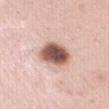Clinical impression: The lesion was tiled from a total-body skin photograph and was not biopsied. Context: Automated tile analysis of the lesion measured a shape-asymmetry score of about 0.2 (0 = symmetric). The software also gave a mean CIELAB color near L≈55 a*≈21 b*≈24 and a normalized border contrast of about 13. The software also gave a border-irregularity rating of about 2/10 and internal color variation of about 6.5 on a 0–10 scale. The analysis additionally found a classifier nevus-likeness of about 90/100. A roughly 15 mm field-of-view crop from a total-body skin photograph. Captured under white-light illumination. The lesion is located on the chest. The recorded lesion diameter is about 4 mm. A female subject in their 50s.